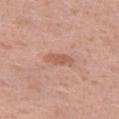| key | value |
|---|---|
| workup | catalogued during a skin exam; not biopsied |
| anatomic site | the left thigh |
| illumination | white-light |
| patient | female, approximately 40 years of age |
| lesion size | about 3 mm |
| acquisition | ~15 mm tile from a whole-body skin photo |